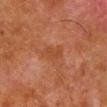Case summary:
– notes · imaged on a skin check; not biopsied
– automated metrics · a footprint of about 5 mm², an eccentricity of roughly 0.75, and two-axis asymmetry of about 0.45; a mean CIELAB color near L≈36 a*≈22 b*≈29, about 5 CIELAB-L* units darker than the surrounding skin, and a lesion-to-skin contrast of about 5 (normalized; higher = more distinct); a border-irregularity index near 4/10, a within-lesion color-variation index near 1.5/10, and peripheral color asymmetry of about 0.5; a classifier nevus-likeness of about 0/100
– lesion diameter · ~3 mm (longest diameter)
– subject · male, about 80 years old
– illumination · cross-polarized
– acquisition · 15 mm crop, total-body photography
– body site · the right lower leg